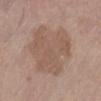Assessment:
This lesion was catalogued during total-body skin photography and was not selected for biopsy.
Context:
Imaged with white-light lighting. A 15 mm close-up tile from a total-body photography series done for melanoma screening. On the left lower leg. A female subject in their mid-60s. Approximately 7.5 mm at its widest.